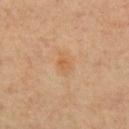| field | value |
|---|---|
| notes | catalogued during a skin exam; not biopsied |
| imaging modality | ~15 mm crop, total-body skin-cancer survey |
| site | the chest |
| lighting | cross-polarized |
| lesion size | about 2.5 mm |
| subject | male, roughly 70 years of age |